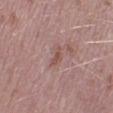Captured during whole-body skin photography for melanoma surveillance; the lesion was not biopsied. The lesion's longest dimension is about 2.5 mm. A roughly 15 mm field-of-view crop from a total-body skin photograph. A male patient approximately 40 years of age. Automated tile analysis of the lesion measured a footprint of about 2.5 mm², an eccentricity of roughly 0.9, and a shape-asymmetry score of about 0.4 (0 = symmetric). And it measured roughly 7 lightness units darker than nearby skin and a normalized border contrast of about 6.5. On the right lower leg. Captured under white-light illumination.